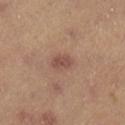workup: catalogued during a skin exam; not biopsied
TBP lesion metrics: a lesion color around L≈48 a*≈21 b*≈24 in CIELAB, about 9 CIELAB-L* units darker than the surrounding skin, and a normalized lesion–skin contrast near 7
patient: female, roughly 35 years of age
lighting: cross-polarized illumination
diameter: about 2.5 mm
imaging modality: ~15 mm crop, total-body skin-cancer survey
site: the right lower leg